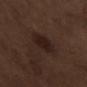Notes:
* biopsy status — imaged on a skin check; not biopsied
* patient — male, aged approximately 70
* anatomic site — the arm
* acquisition — 15 mm crop, total-body photography